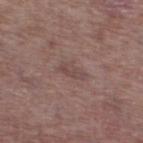No biopsy was performed on this lesion — it was imaged during a full skin examination and was not determined to be concerning. A male subject, aged 73 to 77. This is a white-light tile. Automated image analysis of the tile measured a lesion area of about 4.5 mm², an outline eccentricity of about 0.9 (0 = round, 1 = elongated), and a shape-asymmetry score of about 0.25 (0 = symmetric). The software also gave a mean CIELAB color near L≈46 a*≈17 b*≈20, about 6 CIELAB-L* units darker than the surrounding skin, and a normalized lesion–skin contrast near 5. The software also gave a classifier nevus-likeness of about 0/100. A region of skin cropped from a whole-body photographic capture, roughly 15 mm wide. The lesion is on the left thigh. About 3 mm across.